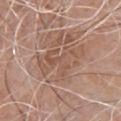biopsy_status: not biopsied; imaged during a skin examination
lesion_size:
  long_diameter_mm_approx: 6.5
automated_metrics:
  area_mm2_approx: 16.0
  vs_skin_darker_L: 7.0
  vs_skin_contrast_norm: 5.0
  border_irregularity_0_10: 7.0
  color_variation_0_10: 4.0
  peripheral_color_asymmetry: 1.5
  nevus_likeness_0_100: 0
  lesion_detection_confidence_0_100: 70
lighting: white-light
image:
  source: total-body photography crop
  field_of_view_mm: 15
patient:
  sex: male
  age_approx: 80
site: chest